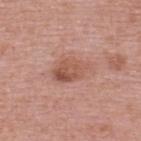follow-up: no biopsy performed (imaged during a skin exam)
image-analysis metrics: a border-irregularity index near 3/10, a color-variation rating of about 6/10, and radial color variation of about 2
subject: female, approximately 60 years of age
lesion size: ~4.5 mm (longest diameter)
location: the upper back
image source: ~15 mm crop, total-body skin-cancer survey